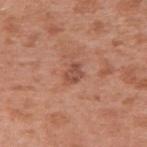Assessment: Recorded during total-body skin imaging; not selected for excision or biopsy. Background: A female patient aged 38 to 42. From the arm. A lesion tile, about 15 mm wide, cut from a 3D total-body photograph.